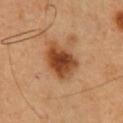Assessment: Captured during whole-body skin photography for melanoma surveillance; the lesion was not biopsied. Context: A male patient, aged 48 to 52. A 15 mm close-up extracted from a 3D total-body photography capture. Measured at roughly 5 mm in maximum diameter. Automated tile analysis of the lesion measured a shape eccentricity near 0.75 and two-axis asymmetry of about 0.2. And it measured an average lesion color of about L≈46 a*≈24 b*≈37 (CIELAB), about 14 CIELAB-L* units darker than the surrounding skin, and a lesion-to-skin contrast of about 10.5 (normalized; higher = more distinct). Located on the chest. Captured under cross-polarized illumination.